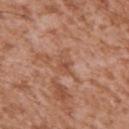Q: Is there a histopathology result?
A: catalogued during a skin exam; not biopsied
Q: What is the imaging modality?
A: ~15 mm tile from a whole-body skin photo
Q: Patient demographics?
A: male, in their mid- to late 40s
Q: Automated lesion metrics?
A: an average lesion color of about L≈52 a*≈23 b*≈32 (CIELAB), roughly 7 lightness units darker than nearby skin, and a normalized lesion–skin contrast near 5.5; an automated nevus-likeness rating near 0 out of 100
Q: Lesion location?
A: the left upper arm